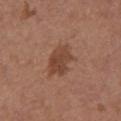The lesion was photographed on a routine skin check and not biopsied; there is no pathology result. A female patient in their mid- to late 60s. On the chest. This is a white-light tile. The recorded lesion diameter is about 4.5 mm. A roughly 15 mm field-of-view crop from a total-body skin photograph.